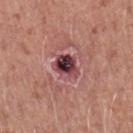{"biopsy_status": "not biopsied; imaged during a skin examination", "site": "left forearm", "lesion_size": {"long_diameter_mm_approx": 3.5}, "patient": {"sex": "male", "age_approx": 50}, "image": {"source": "total-body photography crop", "field_of_view_mm": 15}, "lighting": "white-light", "automated_metrics": {"area_mm2_approx": 6.5, "shape_asymmetry": 0.25, "border_irregularity_0_10": 2.5, "color_variation_0_10": 10.0, "peripheral_color_asymmetry": 3.0}}